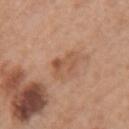The lesion was photographed on a routine skin check and not biopsied; there is no pathology result. A region of skin cropped from a whole-body photographic capture, roughly 15 mm wide. The lesion is located on the right forearm. The subject is a female approximately 60 years of age.